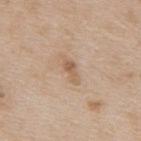Q: Is there a histopathology result?
A: total-body-photography surveillance lesion; no biopsy
Q: Where on the body is the lesion?
A: the upper back
Q: What kind of image is this?
A: ~15 mm crop, total-body skin-cancer survey
Q: Who is the patient?
A: male, roughly 65 years of age